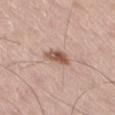No biopsy was performed on this lesion — it was imaged during a full skin examination and was not determined to be concerning. The lesion is on the leg. The patient is a male about 55 years old. Cropped from a total-body skin-imaging series; the visible field is about 15 mm.